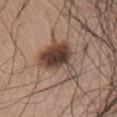notes=catalogued during a skin exam; not biopsied | image source=~15 mm crop, total-body skin-cancer survey | anatomic site=the chest | patient=male, aged approximately 70.